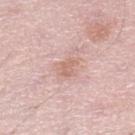The lesion was photographed on a routine skin check and not biopsied; there is no pathology result. Automated image analysis of the tile measured a classifier nevus-likeness of about 5/100 and lesion-presence confidence of about 100/100. Captured under white-light illumination. Longest diameter approximately 3 mm. The lesion is located on the left lower leg. This image is a 15 mm lesion crop taken from a total-body photograph. The subject is a male approximately 50 years of age.